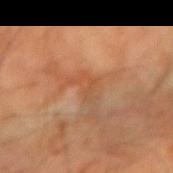The lesion was photographed on a routine skin check and not biopsied; there is no pathology result.
A roughly 15 mm field-of-view crop from a total-body skin photograph.
A male patient, aged around 60.
About 3.5 mm across.
The lesion is on the left forearm.